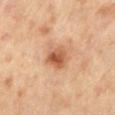No biopsy was performed on this lesion — it was imaged during a full skin examination and was not determined to be concerning. A roughly 15 mm field-of-view crop from a total-body skin photograph. Measured at roughly 3.5 mm in maximum diameter. Captured under cross-polarized illumination. Located on the mid back. A male subject roughly 55 years of age.